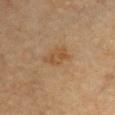The lesion was photographed on a routine skin check and not biopsied; there is no pathology result. The tile uses cross-polarized illumination. The lesion is on the chest. The subject is a female aged approximately 60. A region of skin cropped from a whole-body photographic capture, roughly 15 mm wide. Longest diameter approximately 3.5 mm.